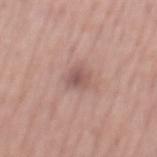Impression: Part of a total-body skin-imaging series; this lesion was reviewed on a skin check and was not flagged for biopsy. Acquisition and patient details: On the mid back. Captured under white-light illumination. A lesion tile, about 15 mm wide, cut from a 3D total-body photograph. A male patient, in their mid- to late 50s. Approximately 2.5 mm at its widest.